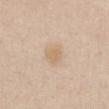| field | value |
|---|---|
| biopsy status | total-body-photography surveillance lesion; no biopsy |